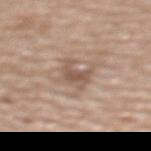Background: The subject is a female in their mid-60s. Located on the upper back. A 15 mm close-up extracted from a 3D total-body photography capture. The lesion-visualizer software estimated an area of roughly 5 mm² and two-axis asymmetry of about 0.5. And it measured a lesion color around L≈54 a*≈17 b*≈26 in CIELAB, roughly 10 lightness units darker than nearby skin, and a normalized lesion–skin contrast near 7. It also reported a nevus-likeness score of about 0/100. Measured at roughly 4 mm in maximum diameter. Captured under white-light illumination.